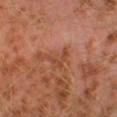follow-up: no biopsy performed (imaged during a skin exam); subject: male, aged 28–32; image source: total-body-photography crop, ~15 mm field of view; site: the left lower leg.